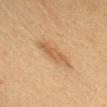Impression:
This lesion was catalogued during total-body skin photography and was not selected for biopsy.
Context:
The lesion is located on the head or neck. The patient is a female aged 18–22. Automated tile analysis of the lesion measured a within-lesion color-variation index near 2/10 and a peripheral color-asymmetry measure near 1. And it measured a nevus-likeness score of about 55/100 and lesion-presence confidence of about 100/100. Cropped from a total-body skin-imaging series; the visible field is about 15 mm. This is a cross-polarized tile. Longest diameter approximately 5 mm.